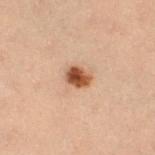biopsy status=no biopsy performed (imaged during a skin exam); site=the right thigh; automated metrics=a border-irregularity index near 1/10 and a color-variation rating of about 4.5/10; patient=female, aged around 40; lesion diameter=~3 mm (longest diameter); imaging modality=total-body-photography crop, ~15 mm field of view; tile lighting=cross-polarized.